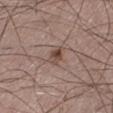Imaged during a routine full-body skin examination; the lesion was not biopsied and no histopathology is available. The lesion is on the left lower leg. The lesion-visualizer software estimated a lesion area of about 3.5 mm², an outline eccentricity of about 0.7 (0 = round, 1 = elongated), and two-axis asymmetry of about 0.25. And it measured a lesion–skin lightness drop of about 10 and a normalized border contrast of about 8. The analysis additionally found a nevus-likeness score of about 80/100 and a detector confidence of about 100 out of 100 that the crop contains a lesion. A 15 mm close-up extracted from a 3D total-body photography capture. A male patient aged around 50. Approximately 2.5 mm at its widest.